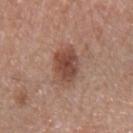Context:
This is a white-light tile. A lesion tile, about 15 mm wide, cut from a 3D total-body photograph. The patient is a female aged 48 to 52. An algorithmic analysis of the crop reported an area of roughly 12 mm². And it measured an average lesion color of about L≈47 a*≈21 b*≈27 (CIELAB) and about 11 CIELAB-L* units darker than the surrounding skin. The analysis additionally found an automated nevus-likeness rating near 90 out of 100 and lesion-presence confidence of about 100/100. Approximately 4.5 mm at its widest. On the right forearm.